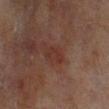workup: total-body-photography surveillance lesion; no biopsy | image source: ~15 mm tile from a whole-body skin photo | patient: female, aged approximately 80 | automated metrics: an average lesion color of about L≈27 a*≈19 b*≈21 (CIELAB), about 5 CIELAB-L* units darker than the surrounding skin, and a normalized border contrast of about 5; a border-irregularity rating of about 5.5/10, internal color variation of about 0 on a 0–10 scale, and a peripheral color-asymmetry measure near 0; a classifier nevus-likeness of about 0/100 | anatomic site: the left lower leg | illumination: cross-polarized illumination | lesion size: about 2.5 mm.